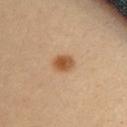Background: The lesion is located on the upper back. A female patient aged approximately 35. A 15 mm close-up extracted from a 3D total-body photography capture.